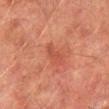biopsy status: imaged on a skin check; not biopsied | site: the leg | TBP lesion metrics: a mean CIELAB color near L≈42 a*≈28 b*≈29, roughly 6 lightness units darker than nearby skin, and a normalized border contrast of about 4.5 | subject: male, aged 73 to 77 | image: ~15 mm crop, total-body skin-cancer survey | lighting: cross-polarized illumination.